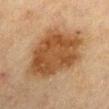biopsy status: no biopsy performed (imaged during a skin exam)
imaging modality: ~15 mm tile from a whole-body skin photo
location: the right upper arm
lighting: cross-polarized
patient: male, aged 68 to 72